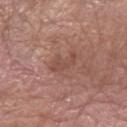A roughly 15 mm field-of-view crop from a total-body skin photograph. A male subject, aged around 60. On the left forearm.